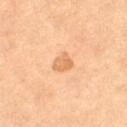biopsy status = total-body-photography surveillance lesion; no biopsy | size = about 2.5 mm | image = total-body-photography crop, ~15 mm field of view | patient = female, aged 63 to 67 | illumination = cross-polarized | body site = the left thigh.